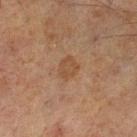follow-up: total-body-photography surveillance lesion; no biopsy
diameter: ≈2.5 mm
body site: the leg
imaging modality: ~15 mm crop, total-body skin-cancer survey
patient: male, about 70 years old